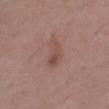Imaged during a routine full-body skin examination; the lesion was not biopsied and no histopathology is available. A 15 mm close-up tile from a total-body photography series done for melanoma screening. An algorithmic analysis of the crop reported a lesion color around L≈49 a*≈19 b*≈25 in CIELAB, a lesion–skin lightness drop of about 7, and a normalized lesion–skin contrast near 6. A male patient aged approximately 75. Approximately 4 mm at its widest. This is a white-light tile. Located on the right thigh.